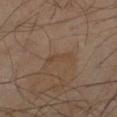Clinical impression: Part of a total-body skin-imaging series; this lesion was reviewed on a skin check and was not flagged for biopsy. Image and clinical context: About 3.5 mm across. A 15 mm crop from a total-body photograph taken for skin-cancer surveillance. The tile uses cross-polarized illumination. The patient is a male approximately 60 years of age. From the right thigh. An algorithmic analysis of the crop reported a lesion area of about 3 mm² and an eccentricity of roughly 0.95. It also reported a lesion color around L≈38 a*≈14 b*≈25 in CIELAB, about 5 CIELAB-L* units darker than the surrounding skin, and a normalized lesion–skin contrast near 4.5. The analysis additionally found an automated nevus-likeness rating near 0 out of 100 and a lesion-detection confidence of about 95/100.